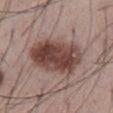| key | value |
|---|---|
| follow-up | imaged on a skin check; not biopsied |
| subject | male, approximately 55 years of age |
| location | the abdomen |
| image | ~15 mm tile from a whole-body skin photo |